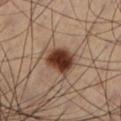Clinical impression:
Imaged during a routine full-body skin examination; the lesion was not biopsied and no histopathology is available.
Context:
On the leg. This is a cross-polarized tile. A lesion tile, about 15 mm wide, cut from a 3D total-body photograph. The patient is a male aged around 65.